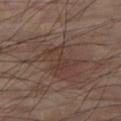Q: Was this lesion biopsied?
A: no biopsy performed (imaged during a skin exam)
Q: Patient demographics?
A: male, aged approximately 60
Q: How was the tile lit?
A: cross-polarized illumination
Q: What is the anatomic site?
A: the right thigh
Q: What kind of image is this?
A: ~15 mm tile from a whole-body skin photo
Q: Lesion size?
A: ~4 mm (longest diameter)
Q: What did automated image analysis measure?
A: lesion-presence confidence of about 100/100